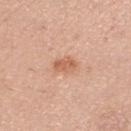Q: Was this lesion biopsied?
A: catalogued during a skin exam; not biopsied
Q: Lesion size?
A: about 3 mm
Q: Who is the patient?
A: female, roughly 30 years of age
Q: What is the imaging modality?
A: ~15 mm crop, total-body skin-cancer survey
Q: What is the anatomic site?
A: the left lower leg
Q: How was the tile lit?
A: white-light illumination